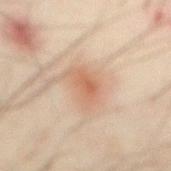Case summary:
• biopsy status — imaged on a skin check; not biopsied
• diameter — ~4 mm (longest diameter)
• acquisition — total-body-photography crop, ~15 mm field of view
• site — the abdomen
• subject — male, about 50 years old
• TBP lesion metrics — a mean CIELAB color near L≈56 a*≈19 b*≈30, roughly 8 lightness units darker than nearby skin, and a lesion-to-skin contrast of about 6 (normalized; higher = more distinct)